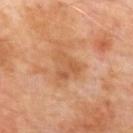Clinical impression:
Captured during whole-body skin photography for melanoma surveillance; the lesion was not biopsied.
Clinical summary:
From the upper back. A male patient about 65 years old. A 15 mm close-up extracted from a 3D total-body photography capture. Imaged with cross-polarized lighting. Approximately 3.5 mm at its widest.